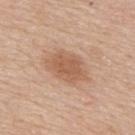Impression: Captured during whole-body skin photography for melanoma surveillance; the lesion was not biopsied. Context: The patient is a male roughly 60 years of age. The lesion's longest dimension is about 5.5 mm. A 15 mm close-up tile from a total-body photography series done for melanoma screening. The lesion is on the upper back.